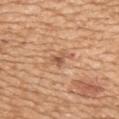| feature | finding |
|---|---|
| follow-up | no biopsy performed (imaged during a skin exam) |